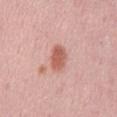biopsy status: total-body-photography surveillance lesion; no biopsy | image: ~15 mm tile from a whole-body skin photo | patient: male, roughly 60 years of age | body site: the abdomen | diameter: about 3.5 mm.